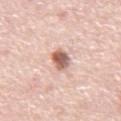Clinical impression:
The lesion was tiled from a total-body skin photograph and was not biopsied.
Acquisition and patient details:
A male subject aged 73–77. A close-up tile cropped from a whole-body skin photograph, about 15 mm across. The recorded lesion diameter is about 3.5 mm. Imaged with white-light lighting. The lesion is on the front of the torso. An algorithmic analysis of the crop reported a footprint of about 5.5 mm² and two-axis asymmetry of about 0.2. The analysis additionally found an average lesion color of about L≈62 a*≈21 b*≈26 (CIELAB), about 17 CIELAB-L* units darker than the surrounding skin, and a normalized border contrast of about 10. The analysis additionally found a classifier nevus-likeness of about 100/100 and a detector confidence of about 100 out of 100 that the crop contains a lesion.